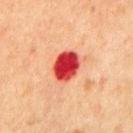Part of a total-body skin-imaging series; this lesion was reviewed on a skin check and was not flagged for biopsy. The recorded lesion diameter is about 3.5 mm. Located on the front of the torso. A male patient, in their mid-60s. A lesion tile, about 15 mm wide, cut from a 3D total-body photograph. Captured under cross-polarized illumination.